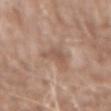Part of a total-body skin-imaging series; this lesion was reviewed on a skin check and was not flagged for biopsy.
This image is a 15 mm lesion crop taken from a total-body photograph.
The lesion is located on the abdomen.
Measured at roughly 3 mm in maximum diameter.
Imaged with white-light lighting.
A male patient, aged around 60.